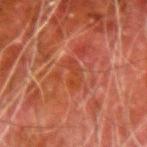follow-up: imaged on a skin check; not biopsied
automated metrics: a lesion color around L≈35 a*≈27 b*≈31 in CIELAB and a lesion-to-skin contrast of about 5 (normalized; higher = more distinct); internal color variation of about 1.5 on a 0–10 scale and a peripheral color-asymmetry measure near 0.5; a classifier nevus-likeness of about 0/100
subject: male, aged around 80
anatomic site: the right forearm
imaging modality: total-body-photography crop, ~15 mm field of view
tile lighting: cross-polarized
size: ≈3.5 mm